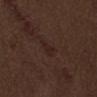Imaged during a routine full-body skin examination; the lesion was not biopsied and no histopathology is available. This is a white-light tile. Located on the mid back. A male subject in their 70s. The lesion's longest dimension is about 2.5 mm. A close-up tile cropped from a whole-body skin photograph, about 15 mm across.